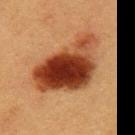Located on the left upper arm. The lesion-visualizer software estimated a footprint of about 31 mm² and a symmetry-axis asymmetry near 0.4. The software also gave an automated nevus-likeness rating near 100 out of 100. Measured at roughly 9 mm in maximum diameter. A male subject roughly 40 years of age. A region of skin cropped from a whole-body photographic capture, roughly 15 mm wide. Captured under cross-polarized illumination.